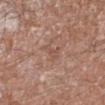{"lighting": "white-light", "lesion_size": {"long_diameter_mm_approx": 2.5}, "patient": {"sex": "male", "age_approx": 60}, "site": "leg", "image": {"source": "total-body photography crop", "field_of_view_mm": 15}, "automated_metrics": {"cielab_L": 52, "cielab_a": 20, "cielab_b": 26, "vs_skin_darker_L": 6.0, "vs_skin_contrast_norm": 4.5, "nevus_likeness_0_100": 0}}